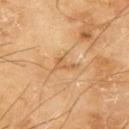Q: Was a biopsy performed?
A: catalogued during a skin exam; not biopsied
Q: How was this image acquired?
A: total-body-photography crop, ~15 mm field of view
Q: How large is the lesion?
A: ≈2.5 mm
Q: What is the anatomic site?
A: the left upper arm
Q: Illumination type?
A: cross-polarized
Q: Who is the patient?
A: male, aged around 65